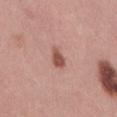Case summary:
- workup — no biopsy performed (imaged during a skin exam)
- site — the lower back
- illumination — white-light
- TBP lesion metrics — a lesion color around L≈53 a*≈24 b*≈27 in CIELAB, a lesion–skin lightness drop of about 12, and a lesion-to-skin contrast of about 8.5 (normalized; higher = more distinct); a within-lesion color-variation index near 2/10 and peripheral color asymmetry of about 0.5; an automated nevus-likeness rating near 95 out of 100 and a detector confidence of about 100 out of 100 that the crop contains a lesion
- imaging modality — 15 mm crop, total-body photography
- subject — female, about 30 years old
- size — ≈2.5 mm